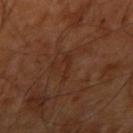{
  "biopsy_status": "not biopsied; imaged during a skin examination",
  "automated_metrics": {
    "nevus_likeness_0_100": 0,
    "lesion_detection_confidence_0_100": 80
  },
  "patient": {
    "age_approx": 65
  },
  "lighting": "cross-polarized",
  "site": "right upper arm",
  "lesion_size": {
    "long_diameter_mm_approx": 3.0
  },
  "image": {
    "source": "total-body photography crop",
    "field_of_view_mm": 15
  }
}